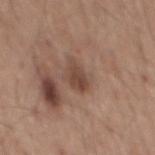Impression: Captured during whole-body skin photography for melanoma surveillance; the lesion was not biopsied. Image and clinical context: From the mid back. Automated image analysis of the tile measured a lesion area of about 5 mm², an outline eccentricity of about 0.75 (0 = round, 1 = elongated), and a shape-asymmetry score of about 0.25 (0 = symmetric). The software also gave an average lesion color of about L≈45 a*≈18 b*≈26 (CIELAB), a lesion–skin lightness drop of about 10, and a normalized border contrast of about 7.5. The software also gave a nevus-likeness score of about 25/100. A region of skin cropped from a whole-body photographic capture, roughly 15 mm wide. A male patient about 55 years old. Imaged with white-light lighting. The lesion's longest dimension is about 3.5 mm.